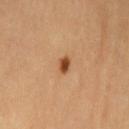Findings:
* biopsy status · total-body-photography surveillance lesion; no biopsy
* location · the left thigh
* patient · female, in their mid- to late 60s
* diameter · ≈2 mm
* image · ~15 mm crop, total-body skin-cancer survey
* tile lighting · cross-polarized